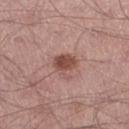Clinical impression: The lesion was tiled from a total-body skin photograph and was not biopsied. Context: About 3 mm across. A male subject aged around 55. Cropped from a total-body skin-imaging series; the visible field is about 15 mm. The tile uses white-light illumination. The lesion is on the left thigh.